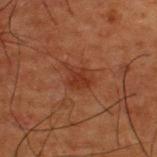Recorded during total-body skin imaging; not selected for excision or biopsy. Measured at roughly 3.5 mm in maximum diameter. A male patient approximately 50 years of age. This is a cross-polarized tile. Cropped from a whole-body photographic skin survey; the tile spans about 15 mm. The lesion is located on the upper back. Automated tile analysis of the lesion measured a footprint of about 6 mm², an outline eccentricity of about 0.65 (0 = round, 1 = elongated), and two-axis asymmetry of about 0.3. It also reported an average lesion color of about L≈26 a*≈21 b*≈26 (CIELAB), roughly 6 lightness units darker than nearby skin, and a normalized lesion–skin contrast near 6.5. The software also gave a lesion-detection confidence of about 100/100.